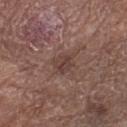Assessment: Captured during whole-body skin photography for melanoma surveillance; the lesion was not biopsied. Clinical summary: Located on the right lower leg. The tile uses white-light illumination. A 15 mm close-up tile from a total-body photography series done for melanoma screening. Longest diameter approximately 2.5 mm. The lesion-visualizer software estimated an average lesion color of about L≈40 a*≈18 b*≈21 (CIELAB), about 7 CIELAB-L* units darker than the surrounding skin, and a normalized lesion–skin contrast near 6.5. And it measured a border-irregularity rating of about 2.5/10 and internal color variation of about 3.5 on a 0–10 scale. The analysis additionally found a nevus-likeness score of about 0/100 and a detector confidence of about 100 out of 100 that the crop contains a lesion. A female subject aged approximately 80.